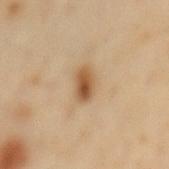No biopsy was performed on this lesion — it was imaged during a full skin examination and was not determined to be concerning.
The lesion is on the mid back.
Cropped from a whole-body photographic skin survey; the tile spans about 15 mm.
Automated tile analysis of the lesion measured lesion-presence confidence of about 100/100.
The tile uses cross-polarized illumination.
A male subject, aged 63 to 67.